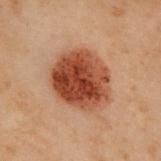follow-up: imaged on a skin check; not biopsied | automated lesion analysis: a lesion area of about 28 mm², an eccentricity of roughly 0.5, and two-axis asymmetry of about 0.15; a lesion color around L≈38 a*≈23 b*≈29 in CIELAB, about 14 CIELAB-L* units darker than the surrounding skin, and a normalized lesion–skin contrast near 11.5; a border-irregularity index near 1.5/10, internal color variation of about 7 on a 0–10 scale, and peripheral color asymmetry of about 2.5; an automated nevus-likeness rating near 100 out of 100 | patient: male, approximately 55 years of age | acquisition: total-body-photography crop, ~15 mm field of view | size: ≈6.5 mm | tile lighting: cross-polarized | body site: the upper back.